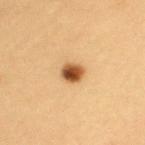Imaged during a routine full-body skin examination; the lesion was not biopsied and no histopathology is available.
The lesion is located on the arm.
Captured under cross-polarized illumination.
A female subject, roughly 30 years of age.
Cropped from a whole-body photographic skin survey; the tile spans about 15 mm.
Measured at roughly 2.5 mm in maximum diameter.